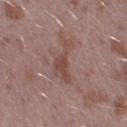Imaged during a routine full-body skin examination; the lesion was not biopsied and no histopathology is available. Automated tile analysis of the lesion measured a lesion color around L≈47 a*≈19 b*≈23 in CIELAB, roughly 8 lightness units darker than nearby skin, and a normalized border contrast of about 6.5. The software also gave a border-irregularity rating of about 7.5/10, a color-variation rating of about 2.5/10, and radial color variation of about 0.5. About 5 mm across. This image is a 15 mm lesion crop taken from a total-body photograph. The subject is a male approximately 40 years of age. On the left lower leg. Captured under white-light illumination.